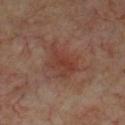<lesion>
  <patient>
    <sex>male</sex>
    <age_approx>70</age_approx>
  </patient>
  <lesion_size>
    <long_diameter_mm_approx>4.5</long_diameter_mm_approx>
  </lesion_size>
  <site>chest</site>
  <automated_metrics>
    <area_mm2_approx>10.0</area_mm2_approx>
    <shape_asymmetry>0.3</shape_asymmetry>
  </automated_metrics>
  <lighting>cross-polarized</lighting>
  <image>
    <source>total-body photography crop</source>
    <field_of_view_mm>15</field_of_view_mm>
  </image>
</lesion>